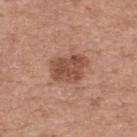Recorded during total-body skin imaging; not selected for excision or biopsy. Automated tile analysis of the lesion measured a mean CIELAB color near L≈50 a*≈23 b*≈30. It also reported a color-variation rating of about 3.5/10 and peripheral color asymmetry of about 1.5. The analysis additionally found an automated nevus-likeness rating near 20 out of 100. The subject is a male approximately 80 years of age. The lesion is on the upper back. A 15 mm crop from a total-body photograph taken for skin-cancer surveillance. This is a white-light tile.